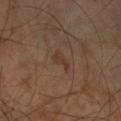Assessment: Captured during whole-body skin photography for melanoma surveillance; the lesion was not biopsied. Clinical summary: The lesion is on the right forearm. A lesion tile, about 15 mm wide, cut from a 3D total-body photograph. Longest diameter approximately 2.5 mm. A male subject in their 70s. An algorithmic analysis of the crop reported an area of roughly 2.5 mm² and a shape-asymmetry score of about 0.45 (0 = symmetric). It also reported a mean CIELAB color near L≈34 a*≈17 b*≈26, a lesion–skin lightness drop of about 6, and a lesion-to-skin contrast of about 6.5 (normalized; higher = more distinct). It also reported a nevus-likeness score of about 15/100 and a lesion-detection confidence of about 100/100. This is a cross-polarized tile.